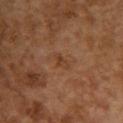Case summary:
– follow-up: total-body-photography surveillance lesion; no biopsy
– subject: female, roughly 60 years of age
– lesion diameter: ≈2.5 mm
– imaging modality: total-body-photography crop, ~15 mm field of view
– location: the back